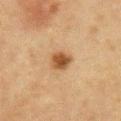Case summary:
• notes · imaged on a skin check; not biopsied
• location · the chest
• image · ~15 mm tile from a whole-body skin photo
• subject · male, aged around 65
• automated lesion analysis · a symmetry-axis asymmetry near 0.25
• lesion diameter · about 3 mm
• illumination · cross-polarized